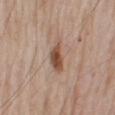The lesion was tiled from a total-body skin photograph and was not biopsied. A male patient roughly 75 years of age. From the right upper arm. A lesion tile, about 15 mm wide, cut from a 3D total-body photograph.